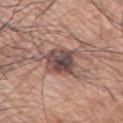Impression: Captured during whole-body skin photography for melanoma surveillance; the lesion was not biopsied. Image and clinical context: A close-up tile cropped from a whole-body skin photograph, about 15 mm across. This is a white-light tile. Located on the left arm. The lesion's longest dimension is about 4.5 mm. The subject is a male aged approximately 70.